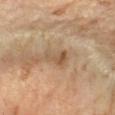The lesion was tiled from a total-body skin photograph and was not biopsied. A close-up tile cropped from a whole-body skin photograph, about 15 mm across. The patient is a female aged around 60. This is a cross-polarized tile. On the left forearm.